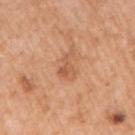Acquisition and patient details: The lesion is located on the arm. Automated image analysis of the tile measured a mean CIELAB color near L≈58 a*≈24 b*≈36, about 8 CIELAB-L* units darker than the surrounding skin, and a lesion-to-skin contrast of about 5.5 (normalized; higher = more distinct). The software also gave an automated nevus-likeness rating near 15 out of 100 and a lesion-detection confidence of about 100/100. A 15 mm crop from a total-body photograph taken for skin-cancer surveillance. The patient is a female aged around 55. The tile uses white-light illumination.